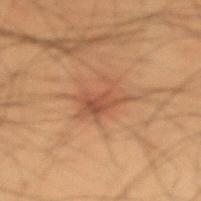Part of a total-body skin-imaging series; this lesion was reviewed on a skin check and was not flagged for biopsy.
A male patient, about 50 years old.
A 15 mm crop from a total-body photograph taken for skin-cancer surveillance.
The total-body-photography lesion software estimated a lesion area of about 8 mm², an eccentricity of roughly 0.8, and a shape-asymmetry score of about 0.4 (0 = symmetric). And it measured a nevus-likeness score of about 30/100 and a detector confidence of about 100 out of 100 that the crop contains a lesion.
Captured under cross-polarized illumination.
On the right forearm.
About 4.5 mm across.